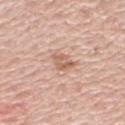patient — female, in their 70s
lesion size — ≈2.5 mm
acquisition — total-body-photography crop, ~15 mm field of view
lighting — white-light illumination
automated metrics — an outline eccentricity of about 0.6 (0 = round, 1 = elongated) and a symmetry-axis asymmetry near 0.3; an automated nevus-likeness rating near 5 out of 100
site — the arm